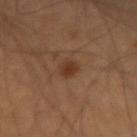notes — total-body-photography surveillance lesion; no biopsy | anatomic site — the left forearm | subject — male, aged 48–52 | lesion size — ~2.5 mm (longest diameter) | lighting — cross-polarized illumination | image source — 15 mm crop, total-body photography.